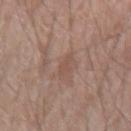{
  "biopsy_status": "not biopsied; imaged during a skin examination",
  "lesion_size": {
    "long_diameter_mm_approx": 3.0
  },
  "patient": {
    "sex": "male",
    "age_approx": 50
  },
  "image": {
    "source": "total-body photography crop",
    "field_of_view_mm": 15
  },
  "site": "right upper arm"
}